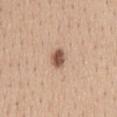Q: Was this lesion biopsied?
A: total-body-photography surveillance lesion; no biopsy
Q: How was the tile lit?
A: white-light illumination
Q: Automated lesion metrics?
A: a mean CIELAB color near L≈54 a*≈20 b*≈28, roughly 16 lightness units darker than nearby skin, and a normalized border contrast of about 10.5; border irregularity of about 2.5 on a 0–10 scale and a within-lesion color-variation index near 3.5/10
Q: What kind of image is this?
A: total-body-photography crop, ~15 mm field of view
Q: What is the lesion's diameter?
A: about 2.5 mm
Q: Lesion location?
A: the back
Q: Patient demographics?
A: male, aged 28 to 32